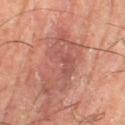Q: How was the tile lit?
A: cross-polarized illumination
Q: Who is the patient?
A: male, aged around 55
Q: Where on the body is the lesion?
A: the leg
Q: How large is the lesion?
A: ≈7.5 mm
Q: What is the imaging modality?
A: ~15 mm tile from a whole-body skin photo